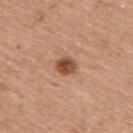• size: ≈2.5 mm
• body site: the upper back
• illumination: white-light illumination
• image: ~15 mm tile from a whole-body skin photo
• patient: male, aged approximately 55